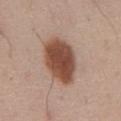* workup — imaged on a skin check; not biopsied
* lesion size — ≈6 mm
* site — the abdomen
* tile lighting — white-light
* patient — male, approximately 60 years of age
* automated lesion analysis — a footprint of about 18 mm² and two-axis asymmetry of about 0.1; an average lesion color of about L≈48 a*≈21 b*≈28 (CIELAB), roughly 17 lightness units darker than nearby skin, and a lesion-to-skin contrast of about 12 (normalized; higher = more distinct); a border-irregularity rating of about 1.5/10
* acquisition — 15 mm crop, total-body photography